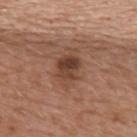Q: Is there a histopathology result?
A: catalogued during a skin exam; not biopsied
Q: Automated lesion metrics?
A: a nevus-likeness score of about 55/100
Q: What are the patient's age and sex?
A: female, aged around 60
Q: Where on the body is the lesion?
A: the back
Q: How large is the lesion?
A: ~4.5 mm (longest diameter)
Q: Illumination type?
A: white-light illumination
Q: What is the imaging modality?
A: ~15 mm crop, total-body skin-cancer survey